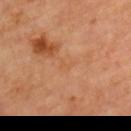Context: The lesion is on the left upper arm. Captured under cross-polarized illumination. A region of skin cropped from a whole-body photographic capture, roughly 15 mm wide. The subject is a female approximately 50 years of age. Automated tile analysis of the lesion measured a footprint of about 1.5 mm², an outline eccentricity of about 0.75 (0 = round, 1 = elongated), and two-axis asymmetry of about 0.4. The analysis additionally found a border-irregularity rating of about 4/10, a color-variation rating of about 0/10, and a peripheral color-asymmetry measure near 0. And it measured an automated nevus-likeness rating near 0 out of 100 and a detector confidence of about 100 out of 100 that the crop contains a lesion.